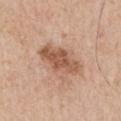acquisition — total-body-photography crop, ~15 mm field of view | lesion size — ~5 mm (longest diameter) | subject — male, in their mid- to late 70s | location — the left upper arm | lighting — white-light | pathology — a melanoma in situ.